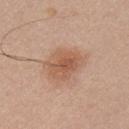No biopsy was performed on this lesion — it was imaged during a full skin examination and was not determined to be concerning. A male subject, aged approximately 30. This is a white-light tile. A close-up tile cropped from a whole-body skin photograph, about 15 mm across. The lesion-visualizer software estimated an average lesion color of about L≈56 a*≈21 b*≈31 (CIELAB), a lesion–skin lightness drop of about 10, and a normalized lesion–skin contrast near 7. It also reported a nevus-likeness score of about 80/100 and lesion-presence confidence of about 100/100. The lesion is located on the chest.